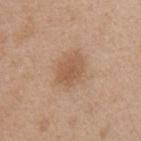Q: Is there a histopathology result?
A: catalogued during a skin exam; not biopsied
Q: What is the imaging modality?
A: 15 mm crop, total-body photography
Q: Lesion location?
A: the back
Q: Who is the patient?
A: female, in their 40s